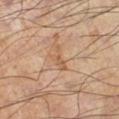| key | value |
|---|---|
| follow-up | total-body-photography surveillance lesion; no biopsy |
| lighting | cross-polarized illumination |
| patient | male, approximately 55 years of age |
| image | ~15 mm crop, total-body skin-cancer survey |
| lesion size | about 3.5 mm |
| body site | the left lower leg |